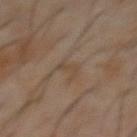• follow-up · imaged on a skin check; not biopsied
• diameter · ≈3 mm
• image · total-body-photography crop, ~15 mm field of view
• location · the abdomen
• patient · male, aged 58–62